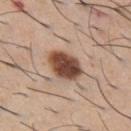Recorded during total-body skin imaging; not selected for excision or biopsy. Located on the chest. This image is a 15 mm lesion crop taken from a total-body photograph. A male subject aged 58–62.